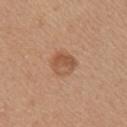Q: Was a biopsy performed?
A: no biopsy performed (imaged during a skin exam)
Q: What are the patient's age and sex?
A: female, aged approximately 45
Q: What is the anatomic site?
A: the right upper arm
Q: How large is the lesion?
A: ~3 mm (longest diameter)
Q: What did automated image analysis measure?
A: roughly 9 lightness units darker than nearby skin; a border-irregularity index near 1.5/10, a within-lesion color-variation index near 5/10, and peripheral color asymmetry of about 2; a detector confidence of about 100 out of 100 that the crop contains a lesion
Q: How was this image acquired?
A: ~15 mm tile from a whole-body skin photo